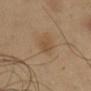| field | value |
|---|---|
| biopsy status | no biopsy performed (imaged during a skin exam) |
| image source | total-body-photography crop, ~15 mm field of view |
| diameter | ~3 mm (longest diameter) |
| subject | male, aged approximately 50 |
| illumination | cross-polarized illumination |
| anatomic site | the chest |